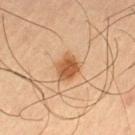Q: What did automated image analysis measure?
A: an area of roughly 6.5 mm², a shape eccentricity near 0.6, and two-axis asymmetry of about 0.2
Q: Where on the body is the lesion?
A: the left thigh
Q: Illumination type?
A: cross-polarized illumination
Q: What is the imaging modality?
A: ~15 mm tile from a whole-body skin photo
Q: Who is the patient?
A: male, roughly 65 years of age
Q: Lesion size?
A: ~3 mm (longest diameter)